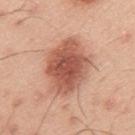  biopsy_status: not biopsied; imaged during a skin examination
  lesion_size:
    long_diameter_mm_approx: 7.0
  patient:
    sex: male
    age_approx: 45
  image:
    source: total-body photography crop
    field_of_view_mm: 15
  lighting: white-light
  site: back